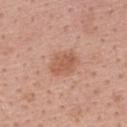notes = no biopsy performed (imaged during a skin exam) | diameter = ≈3 mm | imaging modality = total-body-photography crop, ~15 mm field of view | automated metrics = border irregularity of about 2 on a 0–10 scale, internal color variation of about 2 on a 0–10 scale, and radial color variation of about 1; a nevus-likeness score of about 50/100 and lesion-presence confidence of about 100/100 | tile lighting = white-light illumination | subject = female, aged 33 to 37 | location = the back.